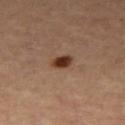Impression:
This lesion was catalogued during total-body skin photography and was not selected for biopsy.
Context:
From the right thigh. The patient is a female roughly 65 years of age. The total-body-photography lesion software estimated a border-irregularity index near 1.5/10 and peripheral color asymmetry of about 1. A 15 mm close-up tile from a total-body photography series done for melanoma screening. The recorded lesion diameter is about 2.5 mm.